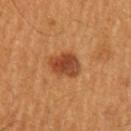Q: Was this lesion biopsied?
A: catalogued during a skin exam; not biopsied